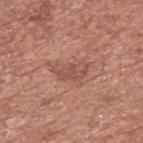• follow-up: no biopsy performed (imaged during a skin exam)
• subject: male, roughly 75 years of age
• site: the upper back
• image: total-body-photography crop, ~15 mm field of view